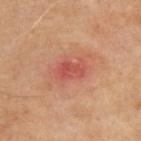The lesion was photographed on a routine skin check and not biopsied; there is no pathology result.
Imaged with cross-polarized lighting.
The lesion is on the upper back.
Longest diameter approximately 3.5 mm.
A 15 mm close-up tile from a total-body photography series done for melanoma screening.
A male subject, in their mid-60s.